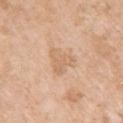Captured during whole-body skin photography for melanoma surveillance; the lesion was not biopsied. Automated tile analysis of the lesion measured a mean CIELAB color near L≈66 a*≈18 b*≈34, about 7 CIELAB-L* units darker than the surrounding skin, and a lesion-to-skin contrast of about 5 (normalized; higher = more distinct). The software also gave a classifier nevus-likeness of about 0/100 and a detector confidence of about 100 out of 100 that the crop contains a lesion. The lesion is located on the arm. Cropped from a total-body skin-imaging series; the visible field is about 15 mm. A female patient, aged approximately 75. This is a white-light tile.